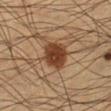Notes:
– notes: total-body-photography surveillance lesion; no biopsy
– tile lighting: cross-polarized
– size: about 3.5 mm
– patient: male, aged around 35
– image: total-body-photography crop, ~15 mm field of view
– anatomic site: the left lower leg